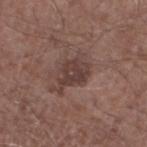Impression:
No biopsy was performed on this lesion — it was imaged during a full skin examination and was not determined to be concerning.
Context:
The total-body-photography lesion software estimated a lesion area of about 7.5 mm², a shape eccentricity near 0.75, and a symmetry-axis asymmetry near 0.4. The analysis additionally found a mean CIELAB color near L≈38 a*≈18 b*≈20, a lesion–skin lightness drop of about 9, and a normalized border contrast of about 8. It also reported a border-irregularity index near 4.5/10 and a peripheral color-asymmetry measure near 1. The analysis additionally found an automated nevus-likeness rating near 0 out of 100 and a detector confidence of about 100 out of 100 that the crop contains a lesion. The subject is a male in their mid-50s. Captured under white-light illumination. A 15 mm close-up extracted from a 3D total-body photography capture. From the leg. About 4 mm across.